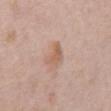A 15 mm crop from a total-body photograph taken for skin-cancer surveillance. The patient is a male about 70 years old. Located on the front of the torso.